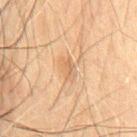Notes:
- notes: total-body-photography surveillance lesion; no biopsy
- subject: male, aged around 60
- imaging modality: 15 mm crop, total-body photography
- lesion diameter: ~3 mm (longest diameter)
- tile lighting: cross-polarized
- automated metrics: an area of roughly 4.5 mm² and an outline eccentricity of about 0.85 (0 = round, 1 = elongated); a classifier nevus-likeness of about 0/100 and a detector confidence of about 100 out of 100 that the crop contains a lesion